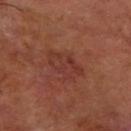Acquisition and patient details: A subject about 65 years old. About 4.5 mm across. On the right forearm. A lesion tile, about 15 mm wide, cut from a 3D total-body photograph. Automated image analysis of the tile measured an average lesion color of about L≈35 a*≈25 b*≈26 (CIELAB) and a lesion–skin lightness drop of about 5. It also reported a within-lesion color-variation index near 3/10 and peripheral color asymmetry of about 1.